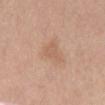Q: Was a biopsy performed?
A: no biopsy performed (imaged during a skin exam)
Q: What is the imaging modality?
A: ~15 mm crop, total-body skin-cancer survey
Q: What is the lesion's diameter?
A: ≈3.5 mm
Q: Lesion location?
A: the abdomen
Q: Patient demographics?
A: female, in their mid-60s